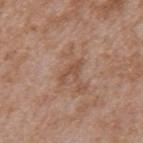Impression:
The lesion was tiled from a total-body skin photograph and was not biopsied.